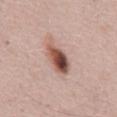- workup · total-body-photography surveillance lesion; no biopsy
- subject · male, aged around 65
- image · ~15 mm crop, total-body skin-cancer survey
- body site · the mid back
- lesion diameter · ≈4.5 mm
- tile lighting · white-light